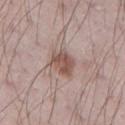biopsy_status: not biopsied; imaged during a skin examination
image:
  source: total-body photography crop
  field_of_view_mm: 15
site: arm
patient:
  sex: male
  age_approx: 70